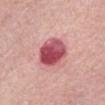workup = total-body-photography surveillance lesion; no biopsy
imaging modality = total-body-photography crop, ~15 mm field of view
patient = male, aged around 65
TBP lesion metrics = a mean CIELAB color near L≈52 a*≈36 b*≈20, about 18 CIELAB-L* units darker than the surrounding skin, and a normalized border contrast of about 11.5; a border-irregularity rating of about 1/10 and a within-lesion color-variation index near 8/10; an automated nevus-likeness rating near 25 out of 100
diameter = ~4.5 mm (longest diameter)
anatomic site = the abdomen
lighting = white-light